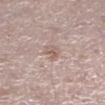Imaged during a routine full-body skin examination; the lesion was not biopsied and no histopathology is available. A roughly 15 mm field-of-view crop from a total-body skin photograph. The lesion is located on the left lower leg. A male subject about 50 years old. Imaged with white-light lighting. About 2.5 mm across.